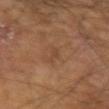Imaged during a routine full-body skin examination; the lesion was not biopsied and no histopathology is available.
Located on the left forearm.
Longest diameter approximately 3 mm.
The patient is a male about 65 years old.
A 15 mm crop from a total-body photograph taken for skin-cancer surveillance.
The lesion-visualizer software estimated a mean CIELAB color near L≈42 a*≈19 b*≈30, roughly 5 lightness units darker than nearby skin, and a normalized border contrast of about 4.5.
Captured under cross-polarized illumination.